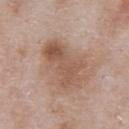The lesion was photographed on a routine skin check and not biopsied; there is no pathology result.
The patient is a male approximately 55 years of age.
On the abdomen.
This image is a 15 mm lesion crop taken from a total-body photograph.
Measured at roughly 6.5 mm in maximum diameter.
The tile uses white-light illumination.